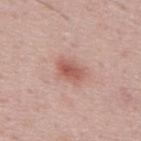Captured during whole-body skin photography for melanoma surveillance; the lesion was not biopsied. A lesion tile, about 15 mm wide, cut from a 3D total-body photograph. A male subject aged 48 to 52. The lesion is on the upper back.